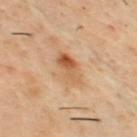{
  "biopsy_status": "not biopsied; imaged during a skin examination",
  "image": {
    "source": "total-body photography crop",
    "field_of_view_mm": 15
  },
  "automated_metrics": {
    "eccentricity": 0.9,
    "shape_asymmetry": 0.2,
    "cielab_L": 47,
    "cielab_a": 18,
    "cielab_b": 31,
    "vs_skin_darker_L": 9.0,
    "vs_skin_contrast_norm": 7.0,
    "border_irregularity_0_10": 2.5,
    "color_variation_0_10": 9.0,
    "nevus_likeness_0_100": 30
  },
  "site": "chest",
  "lesion_size": {
    "long_diameter_mm_approx": 4.5
  },
  "patient": {
    "sex": "male",
    "age_approx": 50
  }
}